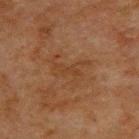follow-up: imaged on a skin check; not biopsied | lighting: cross-polarized illumination | automated metrics: a lesion color around L≈33 a*≈17 b*≈28 in CIELAB, roughly 4 lightness units darker than nearby skin, and a lesion-to-skin contrast of about 5 (normalized; higher = more distinct); a nevus-likeness score of about 0/100 | lesion size: about 4.5 mm | acquisition: 15 mm crop, total-body photography | anatomic site: the upper back | subject: male, roughly 50 years of age.